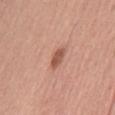Recorded during total-body skin imaging; not selected for excision or biopsy.
A region of skin cropped from a whole-body photographic capture, roughly 15 mm wide.
From the abdomen.
The tile uses white-light illumination.
The lesion's longest dimension is about 3 mm.
A male patient aged 28–32.